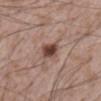notes: no biopsy performed (imaged during a skin exam)
subject: male, approximately 60 years of age
body site: the abdomen
acquisition: 15 mm crop, total-body photography